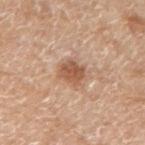This lesion was catalogued during total-body skin photography and was not selected for biopsy. A roughly 15 mm field-of-view crop from a total-body skin photograph. A female patient, aged 73–77. The lesion's longest dimension is about 3 mm. The lesion is on the left forearm.